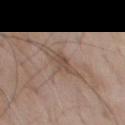notes = imaged on a skin check; not biopsied | patient = male, aged 58–62 | image = total-body-photography crop, ~15 mm field of view | illumination = white-light | location = the chest | automated metrics = a shape eccentricity near 0.8 and a symmetry-axis asymmetry near 0.35 | lesion diameter = about 2.5 mm.